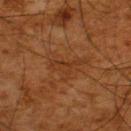<tbp_lesion>
  <biopsy_status>not biopsied; imaged during a skin examination</biopsy_status>
  <image>
    <source>total-body photography crop</source>
    <field_of_view_mm>15</field_of_view_mm>
  </image>
  <automated_metrics>
    <cielab_L>29</cielab_L>
    <cielab_a>19</cielab_a>
    <cielab_b>30</cielab_b>
    <vs_skin_darker_L>5.0</vs_skin_darker_L>
    <vs_skin_contrast_norm>5.0</vs_skin_contrast_norm>
    <border_irregularity_0_10>8.0</border_irregularity_0_10>
    <color_variation_0_10>2.5</color_variation_0_10>
    <peripheral_color_asymmetry>1.0</peripheral_color_asymmetry>
    <nevus_likeness_0_100>0</nevus_likeness_0_100>
    <lesion_detection_confidence_0_100>100</lesion_detection_confidence_0_100>
  </automated_metrics>
  <lesion_size>
    <long_diameter_mm_approx>5.0</long_diameter_mm_approx>
  </lesion_size>
  <patient>
    <sex>male</sex>
    <age_approx>65</age_approx>
  </patient>
  <site>upper back</site>
  <lighting>cross-polarized</lighting>
</tbp_lesion>